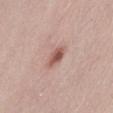Imaged during a routine full-body skin examination; the lesion was not biopsied and no histopathology is available.
A roughly 15 mm field-of-view crop from a total-body skin photograph.
This is a white-light tile.
An algorithmic analysis of the crop reported an outline eccentricity of about 0.85 (0 = round, 1 = elongated) and a symmetry-axis asymmetry near 0.2. And it measured a lesion color around L≈55 a*≈23 b*≈25 in CIELAB, roughly 13 lightness units darker than nearby skin, and a normalized border contrast of about 8.5. The software also gave border irregularity of about 2 on a 0–10 scale, a color-variation rating of about 3.5/10, and radial color variation of about 1.
The patient is a female aged around 30.
The lesion is located on the abdomen.
The recorded lesion diameter is about 3 mm.